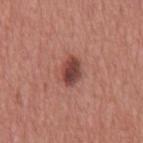This lesion was catalogued during total-body skin photography and was not selected for biopsy. Located on the chest. A 15 mm crop from a total-body photograph taken for skin-cancer surveillance. The patient is a male aged around 45. Captured under white-light illumination. Measured at roughly 3.5 mm in maximum diameter.